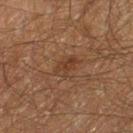Impression: The lesion was tiled from a total-body skin photograph and was not biopsied. Acquisition and patient details: Imaged with cross-polarized lighting. The lesion's longest dimension is about 2.5 mm. A male subject aged approximately 60. A 15 mm close-up extracted from a 3D total-body photography capture. On the left thigh.